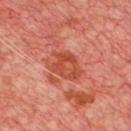Findings:
- workup: no biopsy performed (imaged during a skin exam)
- illumination: cross-polarized illumination
- location: the front of the torso
- automated lesion analysis: an area of roughly 11 mm², an eccentricity of roughly 0.7, and a shape-asymmetry score of about 0.2 (0 = symmetric); roughly 9 lightness units darker than nearby skin and a normalized border contrast of about 7; an automated nevus-likeness rating near 0 out of 100
- lesion diameter: about 4.5 mm
- image source: 15 mm crop, total-body photography
- patient: male, approximately 65 years of age